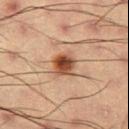Part of a total-body skin-imaging series; this lesion was reviewed on a skin check and was not flagged for biopsy. A close-up tile cropped from a whole-body skin photograph, about 15 mm across. Approximately 3 mm at its widest. Located on the right thigh. This is a cross-polarized tile. An algorithmic analysis of the crop reported a shape eccentricity near 0.5. The analysis additionally found a lesion color around L≈42 a*≈21 b*≈29 in CIELAB, a lesion–skin lightness drop of about 14, and a normalized border contrast of about 11. And it measured a nevus-likeness score of about 95/100 and a lesion-detection confidence of about 100/100. A male patient, in their mid-50s.